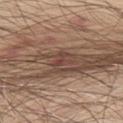Case summary:
- follow-up · no biopsy performed (imaged during a skin exam)
- acquisition · 15 mm crop, total-body photography
- anatomic site · the upper back
- image-analysis metrics · roughly 8 lightness units darker than nearby skin and a normalized border contrast of about 6.5; border irregularity of about 5.5 on a 0–10 scale, a within-lesion color-variation index near 1.5/10, and peripheral color asymmetry of about 0.5; a nevus-likeness score of about 5/100 and lesion-presence confidence of about 65/100
- lighting · white-light illumination
- size · ≈3 mm
- patient · male, aged 63 to 67